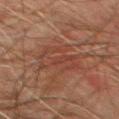workup — no biopsy performed (imaged during a skin exam); site — the front of the torso; tile lighting — cross-polarized; patient — male, in their mid-70s; size — ~6 mm (longest diameter); imaging modality — ~15 mm crop, total-body skin-cancer survey.